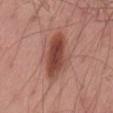Recorded during total-body skin imaging; not selected for excision or biopsy.
A male patient, about 55 years old.
The lesion is on the right thigh.
The tile uses white-light illumination.
A 15 mm close-up tile from a total-body photography series done for melanoma screening.